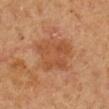Clinical impression:
Recorded during total-body skin imaging; not selected for excision or biopsy.
Image and clinical context:
On the arm. This image is a 15 mm lesion crop taken from a total-body photograph. Captured under cross-polarized illumination. Automated tile analysis of the lesion measured about 7 CIELAB-L* units darker than the surrounding skin and a lesion-to-skin contrast of about 5.5 (normalized; higher = more distinct). Approximately 5 mm at its widest. A male patient, roughly 65 years of age.